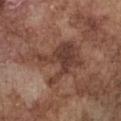This lesion was catalogued during total-body skin photography and was not selected for biopsy. The lesion is located on the chest. The lesion's longest dimension is about 6.5 mm. The tile uses white-light illumination. The lesion-visualizer software estimated an average lesion color of about L≈40 a*≈20 b*≈25 (CIELAB) and a lesion–skin lightness drop of about 9. The analysis additionally found an automated nevus-likeness rating near 0 out of 100 and a detector confidence of about 70 out of 100 that the crop contains a lesion. The patient is a male in their mid- to late 70s. This image is a 15 mm lesion crop taken from a total-body photograph.